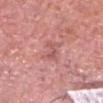Findings:
* follow-up — no biopsy performed (imaged during a skin exam)
* image source — ~15 mm tile from a whole-body skin photo
* patient — male, approximately 80 years of age
* tile lighting — white-light illumination
* body site — the head or neck